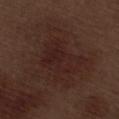Image and clinical context: The subject is a male about 70 years old. This image is a 15 mm lesion crop taken from a total-body photograph. The lesion is on the right thigh.